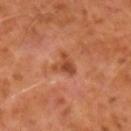This lesion was catalogued during total-body skin photography and was not selected for biopsy.
Located on the arm.
A lesion tile, about 15 mm wide, cut from a 3D total-body photograph.
A male subject, approximately 60 years of age.
Measured at roughly 3.5 mm in maximum diameter.
This is a cross-polarized tile.
Automated tile analysis of the lesion measured an eccentricity of roughly 0.75 and a shape-asymmetry score of about 0.5 (0 = symmetric). The software also gave border irregularity of about 5.5 on a 0–10 scale, a within-lesion color-variation index near 4/10, and radial color variation of about 1.5. It also reported a lesion-detection confidence of about 100/100.